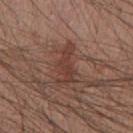| feature | finding |
|---|---|
| notes | total-body-photography surveillance lesion; no biopsy |
| automated lesion analysis | an area of roughly 7.5 mm², a shape eccentricity near 0.9, and a symmetry-axis asymmetry near 0.5; roughly 8 lightness units darker than nearby skin and a normalized border contrast of about 6.5; a classifier nevus-likeness of about 5/100 |
| image source | ~15 mm tile from a whole-body skin photo |
| location | the arm |
| patient | male, aged around 40 |